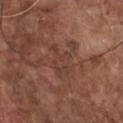| field | value |
|---|---|
| follow-up | catalogued during a skin exam; not biopsied |
| imaging modality | total-body-photography crop, ~15 mm field of view |
| lighting | white-light illumination |
| body site | the chest |
| patient | male, in their mid- to late 70s |